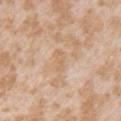Imaged during a routine full-body skin examination; the lesion was not biopsied and no histopathology is available.
A 15 mm close-up extracted from a 3D total-body photography capture.
The lesion-visualizer software estimated a footprint of about 3 mm², a shape eccentricity near 0.9, and two-axis asymmetry of about 0.25. The analysis additionally found a lesion color around L≈65 a*≈17 b*≈34 in CIELAB, roughly 6 lightness units darker than nearby skin, and a lesion-to-skin contrast of about 4.5 (normalized; higher = more distinct). The software also gave border irregularity of about 3 on a 0–10 scale, internal color variation of about 0.5 on a 0–10 scale, and a peripheral color-asymmetry measure near 0. The software also gave an automated nevus-likeness rating near 0 out of 100 and a lesion-detection confidence of about 100/100.
The tile uses white-light illumination.
The patient is a female roughly 25 years of age.
From the right upper arm.
The lesion's longest dimension is about 3 mm.